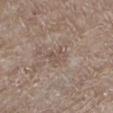Q: Is there a histopathology result?
A: no biopsy performed (imaged during a skin exam)
Q: What kind of image is this?
A: 15 mm crop, total-body photography
Q: Illumination type?
A: white-light
Q: Lesion location?
A: the left lower leg
Q: How large is the lesion?
A: about 2.5 mm
Q: Who is the patient?
A: male, approximately 65 years of age